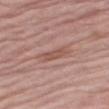workup: no biopsy performed (imaged during a skin exam)
imaging modality: total-body-photography crop, ~15 mm field of view
size: ~3.5 mm (longest diameter)
site: the leg
subject: female, aged 68 to 72
image-analysis metrics: a footprint of about 4.5 mm² and two-axis asymmetry of about 0.3; about 8 CIELAB-L* units darker than the surrounding skin and a lesion-to-skin contrast of about 6 (normalized; higher = more distinct); a nevus-likeness score of about 0/100 and lesion-presence confidence of about 85/100